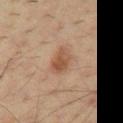lesion_size:
  long_diameter_mm_approx: 3.5
image:
  source: total-body photography crop
  field_of_view_mm: 15
lighting: cross-polarized
patient:
  sex: male
  age_approx: 35
site: chest